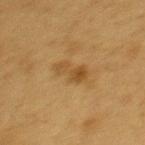{"biopsy_status": "not biopsied; imaged during a skin examination", "site": "back", "patient": {"sex": "male", "age_approx": 55}, "image": {"source": "total-body photography crop", "field_of_view_mm": 15}}